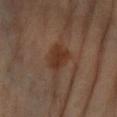biopsy_status: not biopsied; imaged during a skin examination
site: left arm
lesion_size:
  long_diameter_mm_approx: 4.5
lighting: cross-polarized
image:
  source: total-body photography crop
  field_of_view_mm: 15
patient:
  sex: female
  age_approx: 65